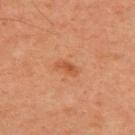  lesion_size:
    long_diameter_mm_approx: 3.0
  image:
    source: total-body photography crop
    field_of_view_mm: 15
  site: chest
  patient:
    sex: male
    age_approx: 60